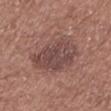follow-up: catalogued during a skin exam; not biopsied
lesion size: about 6.5 mm
body site: the left lower leg
illumination: white-light
image: ~15 mm tile from a whole-body skin photo
subject: male, aged around 70